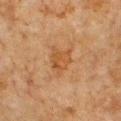Background: The lesion is located on the chest. Captured under cross-polarized illumination. Approximately 3.5 mm at its widest. A male patient about 80 years old. Cropped from a total-body skin-imaging series; the visible field is about 15 mm.